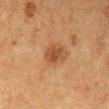biopsy status: no biopsy performed (imaged during a skin exam) | TBP lesion metrics: a lesion color around L≈40 a*≈20 b*≈30 in CIELAB, a lesion–skin lightness drop of about 9, and a lesion-to-skin contrast of about 7 (normalized; higher = more distinct) | anatomic site: the left lower leg | lesion diameter: ≈3.5 mm | tile lighting: cross-polarized illumination | image: total-body-photography crop, ~15 mm field of view | subject: female, roughly 40 years of age.